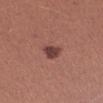Captured during whole-body skin photography for melanoma surveillance; the lesion was not biopsied.
The subject is a female about 25 years old.
A roughly 15 mm field-of-view crop from a total-body skin photograph.
The recorded lesion diameter is about 2.5 mm.
The lesion is on the left forearm.